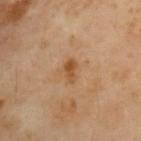* image: ~15 mm crop, total-body skin-cancer survey
* lesion diameter: ≈2.5 mm
* subject: female, aged approximately 60
* location: the upper back
* lighting: cross-polarized